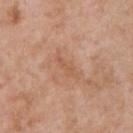follow-up — catalogued during a skin exam; not biopsied
diameter — about 3.5 mm
subject — male, aged approximately 50
TBP lesion metrics — a footprint of about 4 mm², an outline eccentricity of about 0.95 (0 = round, 1 = elongated), and a shape-asymmetry score of about 0.35 (0 = symmetric); border irregularity of about 5 on a 0–10 scale and peripheral color asymmetry of about 0; an automated nevus-likeness rating near 0 out of 100 and lesion-presence confidence of about 100/100
imaging modality — ~15 mm tile from a whole-body skin photo
anatomic site — the front of the torso